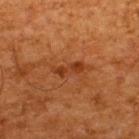Impression: No biopsy was performed on this lesion — it was imaged during a full skin examination and was not determined to be concerning. Context: Imaged with cross-polarized lighting. A male patient approximately 65 years of age. The recorded lesion diameter is about 3.5 mm. The lesion is on the upper back. A 15 mm crop from a total-body photograph taken for skin-cancer surveillance. The total-body-photography lesion software estimated a shape eccentricity near 0.95. And it measured a normalized lesion–skin contrast near 7. And it measured a classifier nevus-likeness of about 0/100 and a lesion-detection confidence of about 100/100.